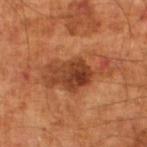Clinical impression: Recorded during total-body skin imaging; not selected for excision or biopsy. Clinical summary: Measured at roughly 7.5 mm in maximum diameter. A 15 mm close-up extracted from a 3D total-body photography capture. The lesion is located on the leg. Captured under cross-polarized illumination. A male patient, roughly 65 years of age.